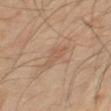Clinical summary:
A close-up tile cropped from a whole-body skin photograph, about 15 mm across. A female patient, approximately 55 years of age. The lesion is located on the left forearm.